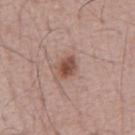Q: Was this lesion biopsied?
A: no biopsy performed (imaged during a skin exam)